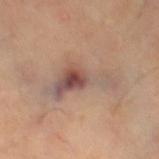biopsy status: imaged on a skin check; not biopsied
lesion size: ≈8 mm
subject: female, about 60 years old
imaging modality: ~15 mm crop, total-body skin-cancer survey
site: the leg
automated lesion analysis: an average lesion color of about L≈54 a*≈17 b*≈24 (CIELAB), a lesion–skin lightness drop of about 9, and a normalized border contrast of about 7; a border-irregularity index near 7/10 and a peripheral color-asymmetry measure near 3